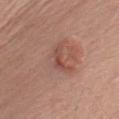Case summary:
– notes · total-body-photography surveillance lesion; no biopsy
– tile lighting · white-light illumination
– image-analysis metrics · a lesion area of about 4 mm² and two-axis asymmetry of about 0.55; a lesion color around L≈48 a*≈24 b*≈26 in CIELAB, a lesion–skin lightness drop of about 8, and a lesion-to-skin contrast of about 6 (normalized; higher = more distinct)
– subject · female, approximately 65 years of age
– body site · the left upper arm
– lesion diameter · about 3.5 mm
– image · ~15 mm tile from a whole-body skin photo